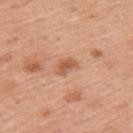Clinical impression: Part of a total-body skin-imaging series; this lesion was reviewed on a skin check and was not flagged for biopsy. Context: The lesion-visualizer software estimated a lesion area of about 4 mm², a shape eccentricity near 0.75, and two-axis asymmetry of about 0.3. The analysis additionally found a mean CIELAB color near L≈58 a*≈25 b*≈35, about 10 CIELAB-L* units darker than the surrounding skin, and a normalized border contrast of about 7. The software also gave a border-irregularity rating of about 3/10, a within-lesion color-variation index near 1.5/10, and a peripheral color-asymmetry measure near 0.5. Located on the left upper arm. Cropped from a whole-body photographic skin survey; the tile spans about 15 mm. A female subject, aged 48–52.